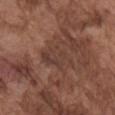follow-up = no biopsy performed (imaged during a skin exam) | subject = male, roughly 75 years of age | site = the right upper arm | image = ~15 mm tile from a whole-body skin photo.